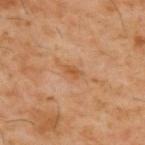Q: Was a biopsy performed?
A: total-body-photography surveillance lesion; no biopsy
Q: How large is the lesion?
A: ~2.5 mm (longest diameter)
Q: Automated lesion metrics?
A: a border-irregularity index near 2.5/10, internal color variation of about 1.5 on a 0–10 scale, and a peripheral color-asymmetry measure near 0.5; an automated nevus-likeness rating near 0 out of 100
Q: Illumination type?
A: cross-polarized
Q: Patient demographics?
A: male, aged approximately 60
Q: Lesion location?
A: the upper back
Q: What is the imaging modality?
A: total-body-photography crop, ~15 mm field of view